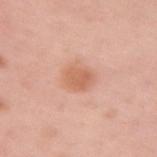| key | value |
|---|---|
| biopsy status | total-body-photography surveillance lesion; no biopsy |
| location | the right upper arm |
| illumination | white-light illumination |
| patient | female, approximately 50 years of age |
| lesion diameter | ≈3 mm |
| image source | 15 mm crop, total-body photography |